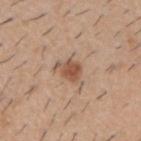Q: Is there a histopathology result?
A: total-body-photography surveillance lesion; no biopsy